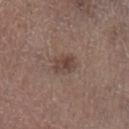Impression: No biopsy was performed on this lesion — it was imaged during a full skin examination and was not determined to be concerning. Context: About 3 mm across. Captured under white-light illumination. An algorithmic analysis of the crop reported a footprint of about 5 mm², a shape eccentricity near 0.7, and a symmetry-axis asymmetry near 0.2. And it measured a lesion color around L≈43 a*≈16 b*≈22 in CIELAB, roughly 9 lightness units darker than nearby skin, and a lesion-to-skin contrast of about 7 (normalized; higher = more distinct). The software also gave border irregularity of about 2.5 on a 0–10 scale and a color-variation rating of about 4/10. It also reported an automated nevus-likeness rating near 15 out of 100 and lesion-presence confidence of about 100/100. Located on the left lower leg. A region of skin cropped from a whole-body photographic capture, roughly 15 mm wide. A male subject, roughly 75 years of age.